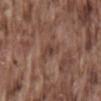biopsy status=catalogued during a skin exam; not biopsied.